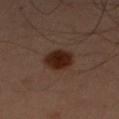<tbp_lesion>
<biopsy_status>not biopsied; imaged during a skin examination</biopsy_status>
<lighting>cross-polarized</lighting>
<image>
  <source>total-body photography crop</source>
  <field_of_view_mm>15</field_of_view_mm>
</image>
<site>arm</site>
<lesion_size>
  <long_diameter_mm_approx>4.0</long_diameter_mm_approx>
</lesion_size>
<patient>
  <sex>male</sex>
  <age_approx>45</age_approx>
</patient>
</tbp_lesion>